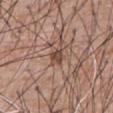follow-up: imaged on a skin check; not biopsied | location: the chest | TBP lesion metrics: an area of roughly 3.5 mm² and an outline eccentricity of about 0.8 (0 = round, 1 = elongated); border irregularity of about 3 on a 0–10 scale, a color-variation rating of about 2.5/10, and a peripheral color-asymmetry measure near 1; an automated nevus-likeness rating near 5 out of 100 | acquisition: ~15 mm tile from a whole-body skin photo | patient: male, approximately 60 years of age | lesion size: ≈3 mm | tile lighting: white-light illumination.